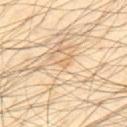biopsy_status: not biopsied; imaged during a skin examination
lighting: cross-polarized
image:
  source: total-body photography crop
  field_of_view_mm: 15
lesion_size:
  long_diameter_mm_approx: 1.0
site: back
patient:
  sex: male
  age_approx: 40